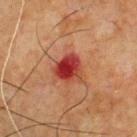image:
  source: total-body photography crop
  field_of_view_mm: 15
patient:
  sex: male
  age_approx: 65
site: front of the torso